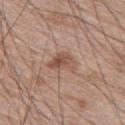biopsy status: imaged on a skin check; not biopsied | acquisition: total-body-photography crop, ~15 mm field of view | automated lesion analysis: a lesion area of about 6 mm² and an outline eccentricity of about 0.75 (0 = round, 1 = elongated); a lesion color around L≈52 a*≈19 b*≈28 in CIELAB, about 10 CIELAB-L* units darker than the surrounding skin, and a normalized border contrast of about 7; a border-irregularity index near 3.5/10 and peripheral color asymmetry of about 1.5; a nevus-likeness score of about 50/100 and a detector confidence of about 100 out of 100 that the crop contains a lesion | patient: male, about 65 years old | illumination: white-light illumination | body site: the back | diameter: ≈3.5 mm.